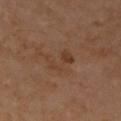Clinical impression:
The lesion was photographed on a routine skin check and not biopsied; there is no pathology result.
Image and clinical context:
A region of skin cropped from a whole-body photographic capture, roughly 15 mm wide. Located on the right upper arm. A female patient, roughly 55 years of age.